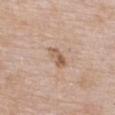A female subject, aged 58 to 62. From the chest. A 15 mm close-up tile from a total-body photography series done for melanoma screening.